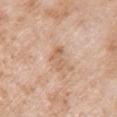Clinical impression: Captured during whole-body skin photography for melanoma surveillance; the lesion was not biopsied. Acquisition and patient details: Cropped from a total-body skin-imaging series; the visible field is about 15 mm. A female subject aged around 70. Located on the left upper arm.